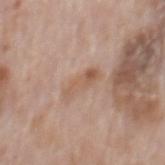site: mid back
image:
  source: total-body photography crop
  field_of_view_mm: 15
patient:
  sex: male
  age_approx: 70